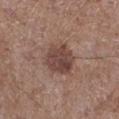<record>
  <biopsy_status>not biopsied; imaged during a skin examination</biopsy_status>
</record>